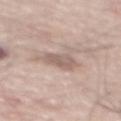biopsy status=catalogued during a skin exam; not biopsied | patient=male, roughly 70 years of age | anatomic site=the back | image source=total-body-photography crop, ~15 mm field of view.